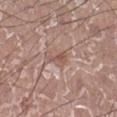Assessment: No biopsy was performed on this lesion — it was imaged during a full skin examination and was not determined to be concerning. Image and clinical context: This image is a 15 mm lesion crop taken from a total-body photograph. The tile uses white-light illumination. The lesion's longest dimension is about 2.5 mm. A male patient, about 50 years old. The lesion is on the right lower leg.